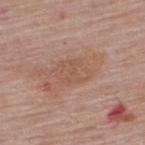The lesion was tiled from a total-body skin photograph and was not biopsied. The lesion is on the upper back. Automated tile analysis of the lesion measured a border-irregularity rating of about 3/10, a within-lesion color-variation index near 2/10, and radial color variation of about 1. A male patient aged 63 to 67. A 15 mm close-up tile from a total-body photography series done for melanoma screening.